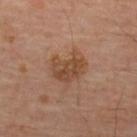Part of a total-body skin-imaging series; this lesion was reviewed on a skin check and was not flagged for biopsy.
A lesion tile, about 15 mm wide, cut from a 3D total-body photograph.
Automated tile analysis of the lesion measured an area of roughly 11 mm², an outline eccentricity of about 0.5 (0 = round, 1 = elongated), and a shape-asymmetry score of about 0.35 (0 = symmetric). The analysis additionally found a lesion color around L≈44 a*≈19 b*≈30 in CIELAB and about 8 CIELAB-L* units darker than the surrounding skin. The analysis additionally found a classifier nevus-likeness of about 15/100 and lesion-presence confidence of about 100/100.
Captured under cross-polarized illumination.
The lesion is on the back.
A male patient roughly 65 years of age.